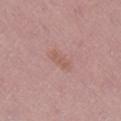{
  "image": {
    "source": "total-body photography crop",
    "field_of_view_mm": 15
  },
  "lighting": "white-light",
  "site": "right lower leg",
  "patient": {
    "sex": "female",
    "age_approx": 50
  },
  "lesion_size": {
    "long_diameter_mm_approx": 3.0
  },
  "automated_metrics": {
    "area_mm2_approx": 4.0,
    "shape_asymmetry": 0.25,
    "cielab_L": 57,
    "cielab_a": 21,
    "cielab_b": 24,
    "vs_skin_contrast_norm": 5.0,
    "nevus_likeness_0_100": 0
  }
}